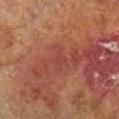This lesion was catalogued during total-body skin photography and was not selected for biopsy. Cropped from a whole-body photographic skin survey; the tile spans about 15 mm. Measured at roughly 3.5 mm in maximum diameter. The lesion is located on the right lower leg. The subject is a male roughly 70 years of age.